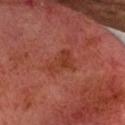Recorded during total-body skin imaging; not selected for excision or biopsy.
A roughly 15 mm field-of-view crop from a total-body skin photograph.
On the head or neck.
Automated tile analysis of the lesion measured an outline eccentricity of about 0.8 (0 = round, 1 = elongated) and a shape-asymmetry score of about 0.4 (0 = symmetric).
The recorded lesion diameter is about 4.5 mm.
A male subject approximately 60 years of age.
Imaged with cross-polarized lighting.